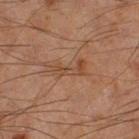follow-up: imaged on a skin check; not biopsied | lesion size: ~4.5 mm (longest diameter) | subject: male, aged approximately 60 | image: ~15 mm tile from a whole-body skin photo | location: the left thigh | image-analysis metrics: an average lesion color of about L≈41 a*≈18 b*≈29 (CIELAB), about 6 CIELAB-L* units darker than the surrounding skin, and a lesion-to-skin contrast of about 6 (normalized; higher = more distinct); a border-irregularity index near 5.5/10 and a within-lesion color-variation index near 3.5/10 | lighting: cross-polarized illumination.